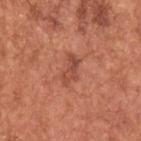The lesion was tiled from a total-body skin photograph and was not biopsied. The patient is a male aged 63 to 67. Automated image analysis of the tile measured a border-irregularity rating of about 5/10, a within-lesion color-variation index near 4/10, and radial color variation of about 1.5. It also reported a classifier nevus-likeness of about 5/100 and a lesion-detection confidence of about 100/100. From the upper back. A region of skin cropped from a whole-body photographic capture, roughly 15 mm wide. Captured under white-light illumination.